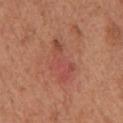workup: imaged on a skin check; not biopsied | lighting: white-light illumination | acquisition: total-body-photography crop, ~15 mm field of view | anatomic site: the chest | subject: male, roughly 65 years of age | automated metrics: a lesion area of about 9.5 mm², an eccentricity of roughly 0.9, and a shape-asymmetry score of about 0.4 (0 = symmetric); a nevus-likeness score of about 0/100.